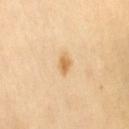Clinical impression: No biopsy was performed on this lesion — it was imaged during a full skin examination and was not determined to be concerning. Background: An algorithmic analysis of the crop reported a footprint of about 3 mm², an eccentricity of roughly 0.7, and a shape-asymmetry score of about 0.35 (0 = symmetric). And it measured an average lesion color of about L≈69 a*≈18 b*≈43 (CIELAB), a lesion–skin lightness drop of about 10, and a normalized lesion–skin contrast near 7.5. The subject is a female aged approximately 70. The recorded lesion diameter is about 2.5 mm. This image is a 15 mm lesion crop taken from a total-body photograph. Located on the right thigh. This is a cross-polarized tile.